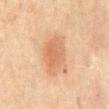workup=no biopsy performed (imaged during a skin exam) | lesion diameter=~4.5 mm (longest diameter) | tile lighting=cross-polarized | subject=male, aged approximately 65 | image source=15 mm crop, total-body photography | automated lesion analysis=a lesion color around L≈51 a*≈19 b*≈30 in CIELAB, roughly 8 lightness units darker than nearby skin, and a normalized border contrast of about 6; border irregularity of about 2 on a 0–10 scale, internal color variation of about 3 on a 0–10 scale, and a peripheral color-asymmetry measure near 1; an automated nevus-likeness rating near 95 out of 100 and a lesion-detection confidence of about 100/100 | location=the front of the torso.